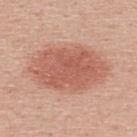Q: Is there a histopathology result?
A: catalogued during a skin exam; not biopsied
Q: What are the patient's age and sex?
A: female, aged 38–42
Q: How large is the lesion?
A: ~9.5 mm (longest diameter)
Q: Where on the body is the lesion?
A: the upper back
Q: What did automated image analysis measure?
A: about 11 CIELAB-L* units darker than the surrounding skin and a lesion-to-skin contrast of about 7 (normalized; higher = more distinct); a classifier nevus-likeness of about 100/100
Q: What kind of image is this?
A: ~15 mm tile from a whole-body skin photo